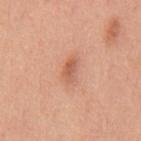workup = total-body-photography surveillance lesion; no biopsy | anatomic site = the mid back | image = ~15 mm crop, total-body skin-cancer survey | diameter = ~2.5 mm (longest diameter) | subject = male, aged 48–52 | lighting = white-light | automated lesion analysis = an average lesion color of about L≈59 a*≈27 b*≈33 (CIELAB) and roughly 10 lightness units darker than nearby skin.